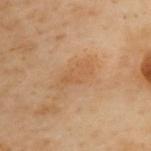The lesion was tiled from a total-body skin photograph and was not biopsied. This is a cross-polarized tile. A female patient, in their 60s. Cropped from a total-body skin-imaging series; the visible field is about 15 mm. Automated image analysis of the tile measured a lesion area of about 6.5 mm², an eccentricity of roughly 0.9, and a symmetry-axis asymmetry near 0.25. And it measured a lesion–skin lightness drop of about 5 and a lesion-to-skin contrast of about 4.5 (normalized; higher = more distinct). The software also gave a border-irregularity rating of about 3/10, internal color variation of about 2 on a 0–10 scale, and radial color variation of about 0.5. The software also gave a detector confidence of about 100 out of 100 that the crop contains a lesion. The lesion is located on the upper back.